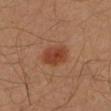Impression:
Part of a total-body skin-imaging series; this lesion was reviewed on a skin check and was not flagged for biopsy.